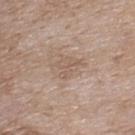notes: no biopsy performed (imaged during a skin exam)
image source: ~15 mm crop, total-body skin-cancer survey
patient: female, in their mid- to late 70s
automated metrics: a mean CIELAB color near L≈57 a*≈15 b*≈26, roughly 7 lightness units darker than nearby skin, and a lesion-to-skin contrast of about 4.5 (normalized; higher = more distinct); a nevus-likeness score of about 0/100 and a lesion-detection confidence of about 100/100
anatomic site: the upper back